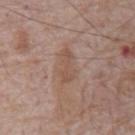Assessment: Recorded during total-body skin imaging; not selected for excision or biopsy. Clinical summary: A 15 mm close-up tile from a total-body photography series done for melanoma screening. This is a white-light tile. From the front of the torso. Automated tile analysis of the lesion measured a border-irregularity index near 6.5/10, a color-variation rating of about 1/10, and peripheral color asymmetry of about 0.5. The software also gave a nevus-likeness score of about 0/100. The subject is a male approximately 70 years of age.